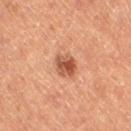Part of a total-body skin-imaging series; this lesion was reviewed on a skin check and was not flagged for biopsy. A female patient aged around 60. This is a cross-polarized tile. A roughly 15 mm field-of-view crop from a total-body skin photograph. From the right thigh. The total-body-photography lesion software estimated a footprint of about 5 mm² and a symmetry-axis asymmetry near 0.2. And it measured a mean CIELAB color near L≈50 a*≈26 b*≈33, about 13 CIELAB-L* units darker than the surrounding skin, and a normalized border contrast of about 9. The software also gave a border-irregularity index near 2/10, internal color variation of about 5.5 on a 0–10 scale, and radial color variation of about 2. The lesion's longest dimension is about 2.5 mm.